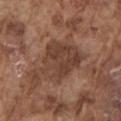Case summary:
* image: ~15 mm crop, total-body skin-cancer survey
* automated metrics: a footprint of about 19 mm² and a shape-asymmetry score of about 0.3 (0 = symmetric); a mean CIELAB color near L≈40 a*≈19 b*≈26, a lesion–skin lightness drop of about 9, and a lesion-to-skin contrast of about 7.5 (normalized; higher = more distinct)
* subject: male, aged approximately 75
* site: the left upper arm
* diameter: about 6 mm
* illumination: white-light illumination